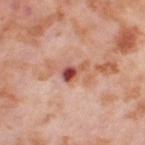Recorded during total-body skin imaging; not selected for excision or biopsy.